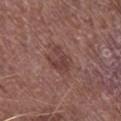Findings:
– anatomic site: the leg
– lighting: white-light
– patient: male, in their mid- to late 70s
– image: 15 mm crop, total-body photography